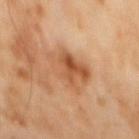• notes — catalogued during a skin exam; not biopsied
• body site — the abdomen
• patient — male, aged 58–62
• lighting — cross-polarized
• lesion diameter — about 4.5 mm
• automated lesion analysis — a lesion area of about 14 mm², an eccentricity of roughly 0.55, and a shape-asymmetry score of about 0.25 (0 = symmetric); a border-irregularity rating of about 3/10 and a within-lesion color-variation index near 8.5/10; a lesion-detection confidence of about 100/100
• imaging modality — total-body-photography crop, ~15 mm field of view